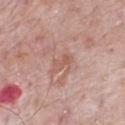biopsy_status: not biopsied; imaged during a skin examination
site: chest
image:
  source: total-body photography crop
  field_of_view_mm: 15
lesion_size:
  long_diameter_mm_approx: 3.0
patient:
  sex: male
  age_approx: 70